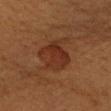notes — total-body-photography surveillance lesion; no biopsy | subject — female, aged 53–57 | image source — ~15 mm tile from a whole-body skin photo | size — about 5 mm | location — the left upper arm | lighting — cross-polarized.